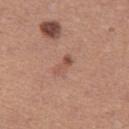Q: Was this lesion biopsied?
A: total-body-photography surveillance lesion; no biopsy
Q: How large is the lesion?
A: about 2.5 mm
Q: What are the patient's age and sex?
A: female, aged around 35
Q: What is the anatomic site?
A: the left thigh
Q: What kind of image is this?
A: ~15 mm crop, total-body skin-cancer survey